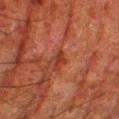Findings:
– follow-up — catalogued during a skin exam; not biopsied
– size — ~2.5 mm (longest diameter)
– patient — male, roughly 80 years of age
– body site — the right thigh
– image — ~15 mm crop, total-body skin-cancer survey